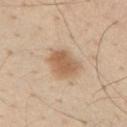follow-up=total-body-photography surveillance lesion; no biopsy | site=the right upper arm | acquisition=~15 mm crop, total-body skin-cancer survey | patient=male, in their mid- to late 50s.